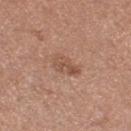The lesion was tiled from a total-body skin photograph and was not biopsied. Located on the right thigh. An algorithmic analysis of the crop reported a nevus-likeness score of about 15/100 and a detector confidence of about 100 out of 100 that the crop contains a lesion. Approximately 3.5 mm at its widest. A region of skin cropped from a whole-body photographic capture, roughly 15 mm wide. A female subject, roughly 30 years of age.